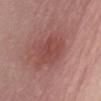Captured during whole-body skin photography for melanoma surveillance; the lesion was not biopsied.
Imaged with white-light lighting.
A 15 mm crop from a total-body photograph taken for skin-cancer surveillance.
A female patient, in their mid-50s.
Approximately 4 mm at its widest.
The lesion is located on the abdomen.